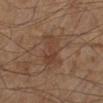  site: right lower leg
  image:
    source: total-body photography crop
    field_of_view_mm: 15
  lesion_size:
    long_diameter_mm_approx: 4.5
  automated_metrics:
    cielab_L: 35
    cielab_a: 16
    cielab_b: 25
    vs_skin_darker_L: 5.0
    vs_skin_contrast_norm: 5.5
    border_irregularity_0_10: 4.0
    color_variation_0_10: 3.0
    peripheral_color_asymmetry: 1.0
  patient:
    sex: male
    age_approx: 60
  lighting: cross-polarized